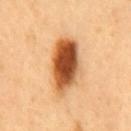Captured under cross-polarized illumination. The total-body-photography lesion software estimated a lesion area of about 20 mm², a shape eccentricity near 0.8, and a symmetry-axis asymmetry near 0.15. And it measured peripheral color asymmetry of about 3. The analysis additionally found an automated nevus-likeness rating near 100 out of 100. A 15 mm crop from a total-body photograph taken for skin-cancer surveillance. Located on the mid back. Longest diameter approximately 6.5 mm. A male subject, aged around 50.